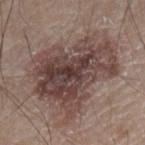{
  "biopsy_status": "not biopsied; imaged during a skin examination",
  "patient": {
    "sex": "male",
    "age_approx": 75
  },
  "lighting": "white-light",
  "image": {
    "source": "total-body photography crop",
    "field_of_view_mm": 15
  },
  "lesion_size": {
    "long_diameter_mm_approx": 10.5
  },
  "site": "left forearm"
}